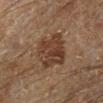biopsy_status: not biopsied; imaged during a skin examination
site: left lower leg
lesion_size:
  long_diameter_mm_approx: 4.5
automated_metrics:
  cielab_L: 31
  cielab_a: 15
  cielab_b: 24
  vs_skin_darker_L: 8.0
  vs_skin_contrast_norm: 8.0
  color_variation_0_10: 4.0
  peripheral_color_asymmetry: 1.5
patient:
  sex: male
  age_approx: 65
image:
  source: total-body photography crop
  field_of_view_mm: 15